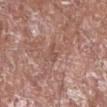Captured during whole-body skin photography for melanoma surveillance; the lesion was not biopsied. The lesion-visualizer software estimated a color-variation rating of about 0.5/10 and radial color variation of about 0. Cropped from a total-body skin-imaging series; the visible field is about 15 mm. The lesion is on the right forearm. A male patient aged approximately 80. The lesion's longest dimension is about 2.5 mm. The tile uses white-light illumination.